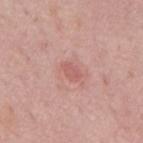Q: Is there a histopathology result?
A: no biopsy performed (imaged during a skin exam)
Q: How was this image acquired?
A: 15 mm crop, total-body photography
Q: Where on the body is the lesion?
A: the mid back
Q: Illumination type?
A: white-light
Q: Patient demographics?
A: male, about 75 years old